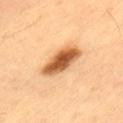Image and clinical context:
An algorithmic analysis of the crop reported border irregularity of about 2 on a 0–10 scale, a within-lesion color-variation index near 6/10, and radial color variation of about 1.5. And it measured a nevus-likeness score of about 100/100 and a detector confidence of about 100 out of 100 that the crop contains a lesion. The subject is a male approximately 60 years of age. The lesion is located on the lower back. A 15 mm close-up tile from a total-body photography series done for melanoma screening. Imaged with cross-polarized lighting.